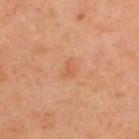Notes:
– workup · imaged on a skin check; not biopsied
– imaging modality · ~15 mm crop, total-body skin-cancer survey
– anatomic site · the back
– patient · female, aged 38–42
– automated metrics · border irregularity of about 2.5 on a 0–10 scale and a within-lesion color-variation index near 1.5/10; a nevus-likeness score of about 0/100 and a detector confidence of about 100 out of 100 that the crop contains a lesion
– tile lighting · cross-polarized